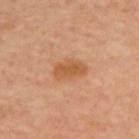biopsy status: imaged on a skin check; not biopsied | image: total-body-photography crop, ~15 mm field of view | lighting: cross-polarized | patient: female, approximately 50 years of age | diameter: ~3.5 mm (longest diameter) | site: the upper back.